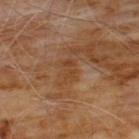Findings:
* notes · imaged on a skin check; not biopsied
* location · the chest
* image source · 15 mm crop, total-body photography
* patient · male, aged approximately 60
* TBP lesion metrics · a footprint of about 3.5 mm², an eccentricity of roughly 0.85, and a symmetry-axis asymmetry near 0.35; a classifier nevus-likeness of about 0/100 and a lesion-detection confidence of about 100/100
* tile lighting · cross-polarized illumination